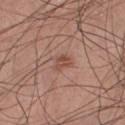<record>
<biopsy_status>not biopsied; imaged during a skin examination</biopsy_status>
<image>
  <source>total-body photography crop</source>
  <field_of_view_mm>15</field_of_view_mm>
</image>
<site>front of the torso</site>
<patient>
  <sex>male</sex>
  <age_approx>30</age_approx>
</patient>
</record>